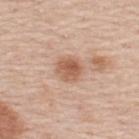Assessment: Recorded during total-body skin imaging; not selected for excision or biopsy. Acquisition and patient details: The tile uses white-light illumination. The lesion's longest dimension is about 3 mm. A male subject, about 60 years old. The lesion is located on the upper back. A 15 mm crop from a total-body photograph taken for skin-cancer surveillance.